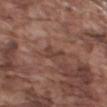Notes:
- notes: imaged on a skin check; not biopsied
- location: the right upper arm
- acquisition: ~15 mm tile from a whole-body skin photo
- subject: male, roughly 75 years of age